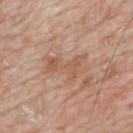{"patient": {"sex": "male", "age_approx": 60}, "site": "mid back", "image": {"source": "total-body photography crop", "field_of_view_mm": 15}, "lesion_size": {"long_diameter_mm_approx": 5.5}}